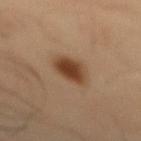Case summary:
- workup — total-body-photography surveillance lesion; no biopsy
- anatomic site — the mid back
- illumination — cross-polarized
- size — ~4 mm (longest diameter)
- imaging modality — 15 mm crop, total-body photography
- subject — male, aged around 55